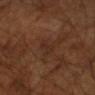Q: Illumination type?
A: cross-polarized
Q: What are the patient's age and sex?
A: male, aged approximately 65
Q: Lesion size?
A: ≈2.5 mm
Q: Automated lesion metrics?
A: border irregularity of about 3 on a 0–10 scale, a color-variation rating of about 0/10, and radial color variation of about 0
Q: How was this image acquired?
A: ~15 mm tile from a whole-body skin photo